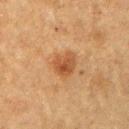The lesion was photographed on a routine skin check and not biopsied; there is no pathology result. The recorded lesion diameter is about 3 mm. A male subject, aged around 75. This is a cross-polarized tile. A lesion tile, about 15 mm wide, cut from a 3D total-body photograph. Located on the right upper arm. An algorithmic analysis of the crop reported an area of roughly 6 mm². The analysis additionally found roughly 9 lightness units darker than nearby skin and a normalized border contrast of about 7.5.